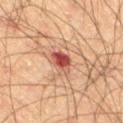workup: catalogued during a skin exam; not biopsied | lighting: cross-polarized | automated lesion analysis: an area of roughly 5.5 mm², an outline eccentricity of about 0.55 (0 = round, 1 = elongated), and two-axis asymmetry of about 0.3; a lesion color around L≈43 a*≈26 b*≈26 in CIELAB and about 13 CIELAB-L* units darker than the surrounding skin; border irregularity of about 3 on a 0–10 scale and a color-variation rating of about 5.5/10 | size: about 3 mm | subject: male, aged 63 to 67 | imaging modality: total-body-photography crop, ~15 mm field of view | site: the right thigh.